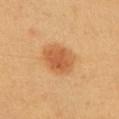Q: Is there a histopathology result?
A: no biopsy performed (imaged during a skin exam)
Q: Where on the body is the lesion?
A: the left upper arm
Q: Patient demographics?
A: female, aged 43–47
Q: What is the imaging modality?
A: ~15 mm crop, total-body skin-cancer survey